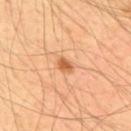Assessment:
Part of a total-body skin-imaging series; this lesion was reviewed on a skin check and was not flagged for biopsy.
Background:
This image is a 15 mm lesion crop taken from a total-body photograph. A male patient aged 53 to 57. An algorithmic analysis of the crop reported an outline eccentricity of about 0.75 (0 = round, 1 = elongated). The analysis additionally found a lesion color around L≈59 a*≈27 b*≈41 in CIELAB, a lesion–skin lightness drop of about 12, and a lesion-to-skin contrast of about 8 (normalized; higher = more distinct). Longest diameter approximately 2 mm. The lesion is located on the upper back.